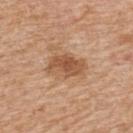This lesion was catalogued during total-body skin photography and was not selected for biopsy.
Imaged with white-light lighting.
About 5 mm across.
From the upper back.
A male subject in their 60s.
A region of skin cropped from a whole-body photographic capture, roughly 15 mm wide.